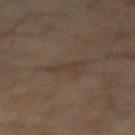Impression: No biopsy was performed on this lesion — it was imaged during a full skin examination and was not determined to be concerning. Acquisition and patient details: On the abdomen. Cropped from a whole-body photographic skin survey; the tile spans about 15 mm. The patient is a male approximately 60 years of age.